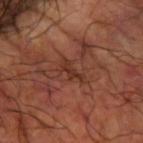No biopsy was performed on this lesion — it was imaged during a full skin examination and was not determined to be concerning.
This image is a 15 mm lesion crop taken from a total-body photograph.
Captured under cross-polarized illumination.
The patient is a male aged 58 to 62.
Located on the right forearm.
Longest diameter approximately 3 mm.